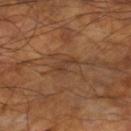No biopsy was performed on this lesion — it was imaged during a full skin examination and was not determined to be concerning.
Approximately 3 mm at its widest.
Cropped from a total-body skin-imaging series; the visible field is about 15 mm.
Located on the right lower leg.
Captured under cross-polarized illumination.
A male subject, aged approximately 60.
Automated tile analysis of the lesion measured an area of roughly 3 mm² and two-axis asymmetry of about 0.5. It also reported a mean CIELAB color near L≈37 a*≈19 b*≈29 and a normalized lesion–skin contrast near 6.